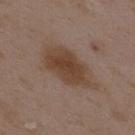{"biopsy_status": "not biopsied; imaged during a skin examination", "image": {"source": "total-body photography crop", "field_of_view_mm": 15}, "lesion_size": {"long_diameter_mm_approx": 7.0}, "lighting": "white-light", "automated_metrics": {"cielab_L": 42, "cielab_a": 16, "cielab_b": 27, "vs_skin_darker_L": 9.0, "vs_skin_contrast_norm": 8.5, "border_irregularity_0_10": 2.5, "nevus_likeness_0_100": 85, "lesion_detection_confidence_0_100": 100}, "patient": {"sex": "female", "age_approx": 35}, "site": "upper back"}